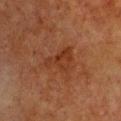No biopsy was performed on this lesion — it was imaged during a full skin examination and was not determined to be concerning. Automated tile analysis of the lesion measured a lesion color around L≈29 a*≈21 b*≈29 in CIELAB and about 5 CIELAB-L* units darker than the surrounding skin. The analysis additionally found a border-irregularity rating of about 6/10, internal color variation of about 3 on a 0–10 scale, and a peripheral color-asymmetry measure near 1. The analysis additionally found a lesion-detection confidence of about 100/100. A female subject approximately 55 years of age. A 15 mm close-up tile from a total-body photography series done for melanoma screening. The lesion is on the chest. Measured at roughly 4.5 mm in maximum diameter.